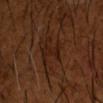<lesion>
<biopsy_status>not biopsied; imaged during a skin examination</biopsy_status>
<automated_metrics>
  <area_mm2_approx>4.5</area_mm2_approx>
  <eccentricity>0.9</eccentricity>
  <shape_asymmetry>0.35</shape_asymmetry>
  <border_irregularity_0_10>4.5</border_irregularity_0_10>
  <nevus_likeness_0_100>0</nevus_likeness_0_100>
</automated_metrics>
<patient>
  <sex>male</sex>
  <age_approx>65</age_approx>
</patient>
<lesion_size>
  <long_diameter_mm_approx>3.5</long_diameter_mm_approx>
</lesion_size>
<image>
  <source>total-body photography crop</source>
  <field_of_view_mm>15</field_of_view_mm>
</image>
<site>left forearm</site>
</lesion>